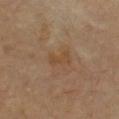follow-up = catalogued during a skin exam; not biopsied.